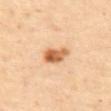Imaged during a routine full-body skin examination; the lesion was not biopsied and no histopathology is available. Cropped from a total-body skin-imaging series; the visible field is about 15 mm. The patient is a female approximately 60 years of age. The lesion is on the upper back. The recorded lesion diameter is about 3.5 mm. The total-body-photography lesion software estimated an area of roughly 6 mm² and an outline eccentricity of about 0.8 (0 = round, 1 = elongated). It also reported an average lesion color of about L≈63 a*≈24 b*≈41 (CIELAB), about 16 CIELAB-L* units darker than the surrounding skin, and a lesion-to-skin contrast of about 10 (normalized; higher = more distinct). And it measured a nevus-likeness score of about 95/100 and a detector confidence of about 100 out of 100 that the crop contains a lesion.